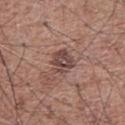Assessment: Part of a total-body skin-imaging series; this lesion was reviewed on a skin check and was not flagged for biopsy. Clinical summary: This is a white-light tile. The patient is a male in their mid-70s. On the chest. Approximately 3.5 mm at its widest. A 15 mm close-up extracted from a 3D total-body photography capture. The total-body-photography lesion software estimated an average lesion color of about L≈45 a*≈19 b*≈21 (CIELAB) and a normalized border contrast of about 8. The software also gave a border-irregularity rating of about 2.5/10, a color-variation rating of about 5/10, and peripheral color asymmetry of about 2. And it measured a lesion-detection confidence of about 100/100.